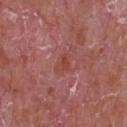biopsy status: catalogued during a skin exam; not biopsied | patient: male, about 65 years old | lesion size: ~2.5 mm (longest diameter) | anatomic site: the chest | tile lighting: white-light illumination | imaging modality: ~15 mm tile from a whole-body skin photo.